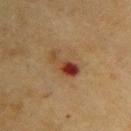notes — no biopsy performed (imaged during a skin exam) | patient — female, aged 53 to 57 | TBP lesion metrics — a mean CIELAB color near L≈36 a*≈22 b*≈29, a lesion–skin lightness drop of about 11, and a normalized border contrast of about 10 | acquisition — ~15 mm crop, total-body skin-cancer survey | size — ≈4 mm | anatomic site — the right upper arm | lighting — cross-polarized.